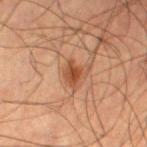Q: Was this lesion biopsied?
A: imaged on a skin check; not biopsied
Q: Where on the body is the lesion?
A: the left thigh
Q: Who is the patient?
A: male, roughly 55 years of age
Q: What is the imaging modality?
A: ~15 mm crop, total-body skin-cancer survey
Q: Lesion size?
A: ~2.5 mm (longest diameter)
Q: Illumination type?
A: cross-polarized